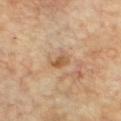{
  "biopsy_status": "not biopsied; imaged during a skin examination",
  "site": "chest",
  "patient": {
    "sex": "male",
    "age_approx": 60
  },
  "image": {
    "source": "total-body photography crop",
    "field_of_view_mm": 15
  },
  "lesion_size": {
    "long_diameter_mm_approx": 2.5
  },
  "lighting": "cross-polarized"
}